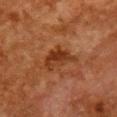| feature | finding |
|---|---|
| biopsy status | total-body-photography surveillance lesion; no biopsy |
| anatomic site | the chest |
| automated metrics | an area of roughly 8 mm² and two-axis asymmetry of about 0.45; a lesion color around L≈29 a*≈22 b*≈31 in CIELAB and about 8 CIELAB-L* units darker than the surrounding skin |
| tile lighting | cross-polarized illumination |
| image | total-body-photography crop, ~15 mm field of view |
| subject | female, roughly 50 years of age |
| lesion diameter | ~4.5 mm (longest diameter) |